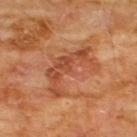{
  "biopsy_status": "not biopsied; imaged during a skin examination",
  "image": {
    "source": "total-body photography crop",
    "field_of_view_mm": 15
  },
  "lighting": "cross-polarized",
  "automated_metrics": {
    "area_mm2_approx": 15.0,
    "cielab_L": 47,
    "cielab_a": 27,
    "cielab_b": 35,
    "vs_skin_contrast_norm": 6.5
  },
  "lesion_size": {
    "long_diameter_mm_approx": 6.5
  },
  "patient": {
    "sex": "male",
    "age_approx": 60
  },
  "site": "chest"
}